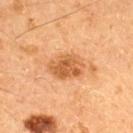Captured during whole-body skin photography for melanoma surveillance; the lesion was not biopsied.
A region of skin cropped from a whole-body photographic capture, roughly 15 mm wide.
Captured under cross-polarized illumination.
Measured at roughly 4 mm in maximum diameter.
A male subject, aged around 60.